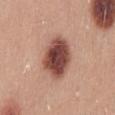<tbp_lesion>
  <patient>
    <sex>male</sex>
    <age_approx>25</age_approx>
  </patient>
  <site>mid back</site>
  <image>
    <source>total-body photography crop</source>
    <field_of_view_mm>15</field_of_view_mm>
  </image>
  <automated_metrics>
    <border_irregularity_0_10>1.5</border_irregularity_0_10>
    <color_variation_0_10>6.5</color_variation_0_10>
    <nevus_likeness_0_100>75</nevus_likeness_0_100>
  </automated_metrics>
  <lesion_size>
    <long_diameter_mm_approx>5.5</long_diameter_mm_approx>
  </lesion_size>
  <lighting>white-light</lighting>
</tbp_lesion>